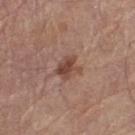Context:
This is a white-light tile. Located on the left thigh. A male patient, in their mid- to late 60s. The lesion-visualizer software estimated an area of roughly 5 mm² and a shape eccentricity near 0.65. And it measured an average lesion color of about L≈45 a*≈21 b*≈26 (CIELAB), a lesion–skin lightness drop of about 11, and a normalized lesion–skin contrast near 8. The analysis additionally found a border-irregularity index near 4/10, internal color variation of about 2.5 on a 0–10 scale, and a peripheral color-asymmetry measure near 0.5. And it measured a classifier nevus-likeness of about 60/100 and lesion-presence confidence of about 100/100. This image is a 15 mm lesion crop taken from a total-body photograph.